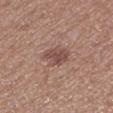No biopsy was performed on this lesion — it was imaged during a full skin examination and was not determined to be concerning.
From the left lower leg.
An algorithmic analysis of the crop reported a lesion area of about 6.5 mm², an eccentricity of roughly 0.55, and two-axis asymmetry of about 0.3. And it measured an average lesion color of about L≈48 a*≈20 b*≈22 (CIELAB) and roughly 10 lightness units darker than nearby skin. The software also gave a color-variation rating of about 2/10 and a peripheral color-asymmetry measure near 0.5.
A female subject, aged approximately 40.
Cropped from a whole-body photographic skin survey; the tile spans about 15 mm.
This is a white-light tile.